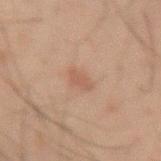Assessment:
Recorded during total-body skin imaging; not selected for excision or biopsy.
Clinical summary:
The tile uses cross-polarized illumination. A male subject aged 33 to 37. The lesion is located on the arm. A roughly 15 mm field-of-view crop from a total-body skin photograph. Automated tile analysis of the lesion measured a footprint of about 4 mm², a shape eccentricity near 0.8, and two-axis asymmetry of about 0.35. And it measured a mean CIELAB color near L≈42 a*≈16 b*≈23, a lesion–skin lightness drop of about 6, and a lesion-to-skin contrast of about 5 (normalized; higher = more distinct). The analysis additionally found an automated nevus-likeness rating near 5 out of 100 and lesion-presence confidence of about 100/100.